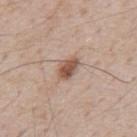Imaged with white-light lighting.
Cropped from a whole-body photographic skin survey; the tile spans about 15 mm.
On the mid back.
Automated image analysis of the tile measured a border-irregularity rating of about 2.5/10. The software also gave a nevus-likeness score of about 95/100 and lesion-presence confidence of about 100/100.
The patient is a male roughly 50 years of age.
The lesion's longest dimension is about 3 mm.